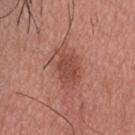Impression: Recorded during total-body skin imaging; not selected for excision or biopsy. Acquisition and patient details: A male subject, aged 38–42. From the head or neck. The lesion-visualizer software estimated about 9 CIELAB-L* units darker than the surrounding skin and a normalized lesion–skin contrast near 6.5. The software also gave a classifier nevus-likeness of about 65/100 and a lesion-detection confidence of about 100/100. This is a white-light tile. Approximately 5.5 mm at its widest. Cropped from a whole-body photographic skin survey; the tile spans about 15 mm.